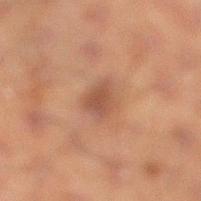Q: Was this lesion biopsied?
A: no biopsy performed (imaged during a skin exam)
Q: Where on the body is the lesion?
A: the right lower leg
Q: How large is the lesion?
A: about 3 mm
Q: Patient demographics?
A: male, aged around 50
Q: What lighting was used for the tile?
A: cross-polarized illumination
Q: How was this image acquired?
A: ~15 mm crop, total-body skin-cancer survey